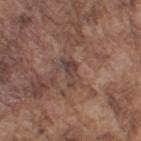biopsy status — no biopsy performed (imaged during a skin exam).